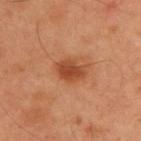Findings:
- workup — catalogued during a skin exam; not biopsied
- site — the upper back
- patient — male, in their mid-50s
- lesion size — ~3.5 mm (longest diameter)
- image — ~15 mm tile from a whole-body skin photo
- tile lighting — cross-polarized illumination